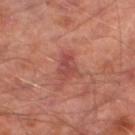– biopsy status: catalogued during a skin exam; not biopsied
– anatomic site: the left lower leg
– image source: ~15 mm crop, total-body skin-cancer survey
– lesion size: ~3.5 mm (longest diameter)
– automated metrics: a shape eccentricity near 0.8 and a shape-asymmetry score of about 0.35 (0 = symmetric); roughly 8 lightness units darker than nearby skin and a lesion-to-skin contrast of about 6.5 (normalized; higher = more distinct); a border-irregularity index near 4/10 and a color-variation rating of about 2/10
– tile lighting: cross-polarized
– patient: male, in their mid-60s